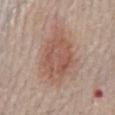The lesion was tiled from a total-body skin photograph and was not biopsied.